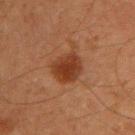This lesion was catalogued during total-body skin photography and was not selected for biopsy.
A male patient about 45 years old.
A close-up tile cropped from a whole-body skin photograph, about 15 mm across.
On the back.